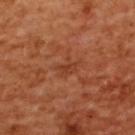  biopsy_status: not biopsied; imaged during a skin examination
  lesion_size:
    long_diameter_mm_approx: 3.0
  image:
    source: total-body photography crop
    field_of_view_mm: 15
  patient:
    sex: female
    age_approx: 55
  site: upper back
  lighting: cross-polarized